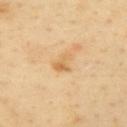• lesion size — ~3 mm (longest diameter)
• subject — male, aged approximately 40
• site — the left upper arm
• image source — total-body-photography crop, ~15 mm field of view